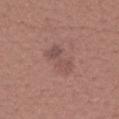Captured during whole-body skin photography for melanoma surveillance; the lesion was not biopsied.
From the head or neck.
Cropped from a total-body skin-imaging series; the visible field is about 15 mm.
A female patient about 30 years old.
Measured at roughly 4 mm in maximum diameter.